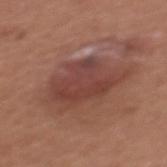This lesion was catalogued during total-body skin photography and was not selected for biopsy. The lesion's longest dimension is about 7.5 mm. The lesion is located on the chest. The total-body-photography lesion software estimated a border-irregularity index near 4.5/10, a within-lesion color-variation index near 4/10, and a peripheral color-asymmetry measure near 1. And it measured an automated nevus-likeness rating near 65 out of 100 and a lesion-detection confidence of about 100/100. This image is a 15 mm lesion crop taken from a total-body photograph. A female subject, approximately 35 years of age.